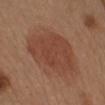Imaged during a routine full-body skin examination; the lesion was not biopsied and no histopathology is available. About 7 mm across. The lesion-visualizer software estimated a nevus-likeness score of about 95/100 and a lesion-detection confidence of about 100/100. On the chest. A female subject aged approximately 40. A 15 mm close-up extracted from a 3D total-body photography capture. Captured under white-light illumination.